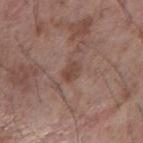Clinical impression: The lesion was tiled from a total-body skin photograph and was not biopsied. Acquisition and patient details: A male subject aged 58 to 62. From the right forearm. The lesion's longest dimension is about 2.5 mm. A close-up tile cropped from a whole-body skin photograph, about 15 mm across. The total-body-photography lesion software estimated a mean CIELAB color near L≈45 a*≈18 b*≈24, a lesion–skin lightness drop of about 8, and a normalized lesion–skin contrast near 6.5. And it measured a classifier nevus-likeness of about 0/100. Captured under white-light illumination.